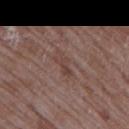body site = the right upper arm; imaging modality = ~15 mm crop, total-body skin-cancer survey; patient = female, in their 70s.